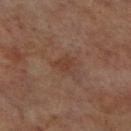Assessment:
This lesion was catalogued during total-body skin photography and was not selected for biopsy.
Background:
On the right lower leg. A male patient in their 70s. Longest diameter approximately 3 mm. Automated image analysis of the tile measured a lesion color around L≈38 a*≈19 b*≈26 in CIELAB and a normalized lesion–skin contrast near 5. It also reported an automated nevus-likeness rating near 0 out of 100. Cropped from a whole-body photographic skin survey; the tile spans about 15 mm. Captured under cross-polarized illumination.